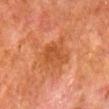workup = catalogued during a skin exam; not biopsied | subject = male, aged around 80 | illumination = cross-polarized | image = ~15 mm crop, total-body skin-cancer survey | lesion diameter = about 4.5 mm | site = the right lower leg.